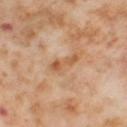Impression:
Imaged during a routine full-body skin examination; the lesion was not biopsied and no histopathology is available.
Clinical summary:
Approximately 3.5 mm at its widest. Imaged with cross-polarized lighting. Automated tile analysis of the lesion measured a footprint of about 4 mm², an eccentricity of roughly 0.95, and two-axis asymmetry of about 0.3. The software also gave a border-irregularity rating of about 4.5/10, a color-variation rating of about 1/10, and peripheral color asymmetry of about 0. And it measured a lesion-detection confidence of about 100/100. The lesion is on the leg. A roughly 15 mm field-of-view crop from a total-body skin photograph. The patient is a female approximately 55 years of age.